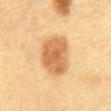location=the chest
patient=female, aged 28–32
image source=total-body-photography crop, ~15 mm field of view